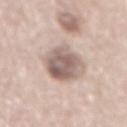Clinical summary: A close-up tile cropped from a whole-body skin photograph, about 15 mm across. On the mid back. A male subject, aged 58–62.